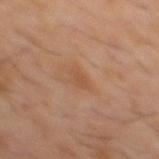The lesion was tiled from a total-body skin photograph and was not biopsied.
Automated image analysis of the tile measured a lesion-detection confidence of about 100/100.
The recorded lesion diameter is about 2.5 mm.
A region of skin cropped from a whole-body photographic capture, roughly 15 mm wide.
This is a cross-polarized tile.
On the back.
A male patient aged around 60.